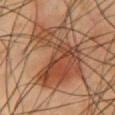follow-up: total-body-photography surveillance lesion; no biopsy | diameter: about 8.5 mm | patient: male, aged around 55 | site: the chest | image: total-body-photography crop, ~15 mm field of view.